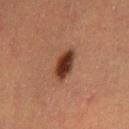Cropped from a whole-body photographic skin survey; the tile spans about 15 mm. The patient is a female approximately 55 years of age. Approximately 4 mm at its widest. The lesion is on the left thigh. Captured under cross-polarized illumination.